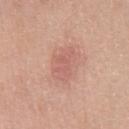| feature | finding |
|---|---|
| workup | catalogued during a skin exam; not biopsied |
| lighting | white-light illumination |
| patient | male, roughly 70 years of age |
| acquisition | ~15 mm crop, total-body skin-cancer survey |
| diameter | ≈4.5 mm |
| location | the chest |